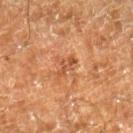| key | value |
|---|---|
| image | total-body-photography crop, ~15 mm field of view |
| location | the right lower leg |
| subject | male, aged 58 to 62 |